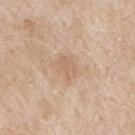Assessment: Recorded during total-body skin imaging; not selected for excision or biopsy. Acquisition and patient details: The lesion-visualizer software estimated an average lesion color of about L≈64 a*≈16 b*≈31 (CIELAB), a lesion–skin lightness drop of about 6, and a lesion-to-skin contrast of about 4.5 (normalized; higher = more distinct). It also reported border irregularity of about 3.5 on a 0–10 scale, a within-lesion color-variation index near 1.5/10, and peripheral color asymmetry of about 0.5. It also reported lesion-presence confidence of about 100/100. The lesion is on the mid back. Imaged with white-light lighting. Cropped from a total-body skin-imaging series; the visible field is about 15 mm. The subject is a male aged 63 to 67.